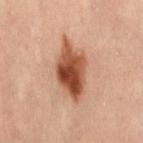follow-up=catalogued during a skin exam; not biopsied.